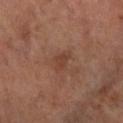follow-up: no biopsy performed (imaged during a skin exam)
diameter: ≈3 mm
lighting: cross-polarized illumination
patient: female, about 65 years old
image source: 15 mm crop, total-body photography
location: the left arm